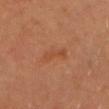Case summary:
* anatomic site: the front of the torso
* lighting: cross-polarized illumination
* automated metrics: an area of roughly 4.5 mm², a shape eccentricity near 0.9, and two-axis asymmetry of about 0.4; an average lesion color of about L≈46 a*≈24 b*≈34 (CIELAB), roughly 5 lightness units darker than nearby skin, and a normalized border contrast of about 5; a within-lesion color-variation index near 2/10 and peripheral color asymmetry of about 1
* imaging modality: 15 mm crop, total-body photography
* patient: male, in their mid-60s
* lesion diameter: about 3.5 mm